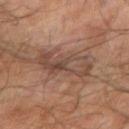No biopsy was performed on this lesion — it was imaged during a full skin examination and was not determined to be concerning. Approximately 6.5 mm at its widest. Located on the arm. A male patient, in their mid-60s. The lesion-visualizer software estimated an area of roughly 13 mm², an eccentricity of roughly 0.95, and a symmetry-axis asymmetry near 0.35. It also reported a lesion color around L≈42 a*≈17 b*≈24 in CIELAB and a normalized border contrast of about 7. And it measured a border-irregularity rating of about 5.5/10 and radial color variation of about 2.5. The analysis additionally found a lesion-detection confidence of about 70/100. Imaged with cross-polarized lighting. This image is a 15 mm lesion crop taken from a total-body photograph.